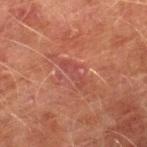Captured during whole-body skin photography for melanoma surveillance; the lesion was not biopsied.